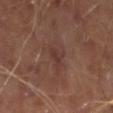• notes: total-body-photography surveillance lesion; no biopsy
• image: ~15 mm crop, total-body skin-cancer survey
• body site: the left lower leg
• subject: male, in their mid-60s
• lesion size: about 2.5 mm
• image-analysis metrics: a mean CIELAB color near L≈34 a*≈19 b*≈21, roughly 6 lightness units darker than nearby skin, and a normalized border contrast of about 5.5; a within-lesion color-variation index near 1.5/10 and peripheral color asymmetry of about 0.5; an automated nevus-likeness rating near 0 out of 100 and a detector confidence of about 100 out of 100 that the crop contains a lesion